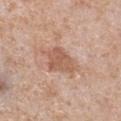Impression: Recorded during total-body skin imaging; not selected for excision or biopsy. Background: From the chest. The subject is a male aged 63 to 67. Imaged with white-light lighting. The total-body-photography lesion software estimated a footprint of about 9 mm², an eccentricity of roughly 0.65, and a symmetry-axis asymmetry near 0.3. It also reported a border-irregularity rating of about 3/10, internal color variation of about 3 on a 0–10 scale, and peripheral color asymmetry of about 1. And it measured an automated nevus-likeness rating near 0 out of 100 and a lesion-detection confidence of about 100/100. A 15 mm crop from a total-body photograph taken for skin-cancer surveillance.